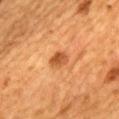biopsy_status: not biopsied; imaged during a skin examination
patient:
  sex: female
  age_approx: 55
site: mid back
image:
  source: total-body photography crop
  field_of_view_mm: 15